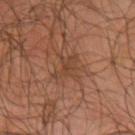subject = male, aged 63 to 67; illumination = cross-polarized; lesion size = about 3 mm; location = the left upper arm; image source = ~15 mm crop, total-body skin-cancer survey.